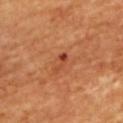The lesion was tiled from a total-body skin photograph and was not biopsied.
The recorded lesion diameter is about 2.5 mm.
The patient is a male in their mid-60s.
A 15 mm crop from a total-body photograph taken for skin-cancer surveillance.
The lesion is located on the upper back.
Automated image analysis of the tile measured an area of roughly 2 mm², a shape eccentricity near 0.9, and a shape-asymmetry score of about 0.5 (0 = symmetric). The software also gave a mean CIELAB color near L≈46 a*≈32 b*≈37, a lesion–skin lightness drop of about 9, and a normalized lesion–skin contrast near 7.